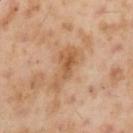Context:
Measured at roughly 5.5 mm in maximum diameter. Captured under cross-polarized illumination. A male subject approximately 55 years of age. A 15 mm close-up tile from a total-body photography series done for melanoma screening. The lesion is on the left upper arm.